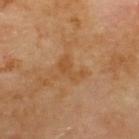Impression: Recorded during total-body skin imaging; not selected for excision or biopsy. Acquisition and patient details: Captured under cross-polarized illumination. The lesion's longest dimension is about 3 mm. The lesion is located on the upper back. This image is a 15 mm lesion crop taken from a total-body photograph. A male subject aged 68 to 72.